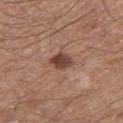notes: total-body-photography surveillance lesion; no biopsy | patient: male, about 65 years old | automated lesion analysis: an area of roughly 5 mm² and an eccentricity of roughly 0.7; a lesion color around L≈43 a*≈21 b*≈25 in CIELAB, roughly 14 lightness units darker than nearby skin, and a normalized border contrast of about 10.5 | lesion diameter: ≈3 mm | location: the left thigh | image source: total-body-photography crop, ~15 mm field of view.